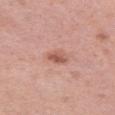Clinical impression:
No biopsy was performed on this lesion — it was imaged during a full skin examination and was not determined to be concerning.
Background:
A 15 mm close-up tile from a total-body photography series done for melanoma screening. The lesion is located on the left thigh. A female patient, in their 40s.